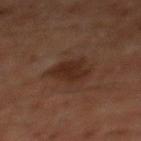Part of a total-body skin-imaging series; this lesion was reviewed on a skin check and was not flagged for biopsy. The subject is a male approximately 60 years of age. Cropped from a total-body skin-imaging series; the visible field is about 15 mm. On the chest.